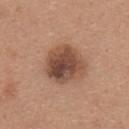notes = no biopsy performed (imaged during a skin exam); anatomic site = the upper back; image = 15 mm crop, total-body photography; patient = female, roughly 35 years of age; diameter = about 5 mm.